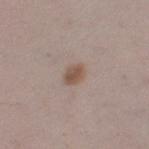Image and clinical context:
A region of skin cropped from a whole-body photographic capture, roughly 15 mm wide. Automated tile analysis of the lesion measured a nevus-likeness score of about 90/100. On the left thigh. A female subject, aged around 20.